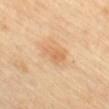Recorded during total-body skin imaging; not selected for excision or biopsy. This is a cross-polarized tile. An algorithmic analysis of the crop reported an area of roughly 7 mm², a shape eccentricity near 0.8, and a symmetry-axis asymmetry near 0.3. The lesion's longest dimension is about 3.5 mm. A 15 mm crop from a total-body photograph taken for skin-cancer surveillance. A female subject, aged approximately 60. The lesion is on the back.